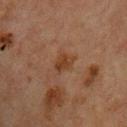notes: catalogued during a skin exam; not biopsied
illumination: cross-polarized
diameter: ≈2.5 mm
anatomic site: the upper back
subject: male, in their mid-60s
image source: total-body-photography crop, ~15 mm field of view
image-analysis metrics: a footprint of about 4 mm², an outline eccentricity of about 0.6 (0 = round, 1 = elongated), and two-axis asymmetry of about 0.3; a mean CIELAB color near L≈31 a*≈17 b*≈27 and about 6 CIELAB-L* units darker than the surrounding skin